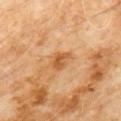Notes:
* workup: no biopsy performed (imaged during a skin exam)
* site: the chest
* patient: male, in their 60s
* lighting: cross-polarized illumination
* imaging modality: ~15 mm tile from a whole-body skin photo
* lesion diameter: ~2.5 mm (longest diameter)